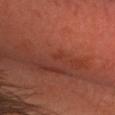follow-up: imaged on a skin check; not biopsied | image: ~15 mm crop, total-body skin-cancer survey | subject: male, in their mid- to late 40s | lesion size: ~1 mm (longest diameter) | TBP lesion metrics: a within-lesion color-variation index near 0/10 and radial color variation of about 0; an automated nevus-likeness rating near 0 out of 100 and lesion-presence confidence of about 95/100 | body site: the head or neck | illumination: cross-polarized illumination.